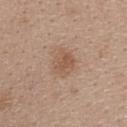The lesion was photographed on a routine skin check and not biopsied; there is no pathology result.
A roughly 15 mm field-of-view crop from a total-body skin photograph.
The lesion is located on the upper back.
The subject is a female roughly 25 years of age.